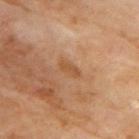{"biopsy_status": "not biopsied; imaged during a skin examination", "patient": {"sex": "male", "age_approx": 70}, "lesion_size": {"long_diameter_mm_approx": 3.0}, "image": {"source": "total-body photography crop", "field_of_view_mm": 15}, "lighting": "cross-polarized", "automated_metrics": {"cielab_L": 51, "cielab_a": 22, "cielab_b": 36, "vs_skin_darker_L": 7.0, "vs_skin_contrast_norm": 6.0, "border_irregularity_0_10": 3.5}, "site": "upper back"}